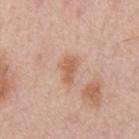Notes:
– biopsy status: catalogued during a skin exam; not biopsied
– lesion size: about 3 mm
– site: the back
– automated metrics: a lesion area of about 5 mm², a shape eccentricity near 0.8, and two-axis asymmetry of about 0.3; a lesion color around L≈61 a*≈21 b*≈32 in CIELAB, a lesion–skin lightness drop of about 9, and a normalized lesion–skin contrast near 6.5
– tile lighting: white-light
– image: ~15 mm crop, total-body skin-cancer survey
– patient: male, in their mid-60s